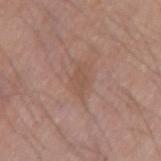follow-up = total-body-photography surveillance lesion; no biopsy | body site = the left upper arm | subject = male, in their mid-40s | image = total-body-photography crop, ~15 mm field of view.